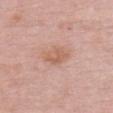Imaged during a routine full-body skin examination; the lesion was not biopsied and no histopathology is available. A female patient, aged around 65. Located on the chest. A lesion tile, about 15 mm wide, cut from a 3D total-body photograph.